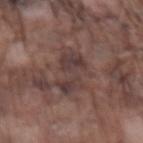Part of a total-body skin-imaging series; this lesion was reviewed on a skin check and was not flagged for biopsy.
A lesion tile, about 15 mm wide, cut from a 3D total-body photograph.
On the left forearm.
A male patient approximately 75 years of age.